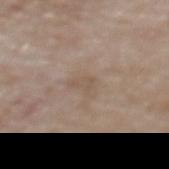Imaged during a routine full-body skin examination; the lesion was not biopsied and no histopathology is available. Located on the upper back. Approximately 3 mm at its widest. Cropped from a total-body skin-imaging series; the visible field is about 15 mm. A female subject aged 73–77. Captured under white-light illumination.